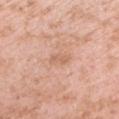workup: catalogued during a skin exam; not biopsied